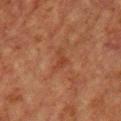Part of a total-body skin-imaging series; this lesion was reviewed on a skin check and was not flagged for biopsy. This is a cross-polarized tile. Longest diameter approximately 3 mm. A male patient, about 60 years old. Located on the chest. A 15 mm close-up tile from a total-body photography series done for melanoma screening. The lesion-visualizer software estimated an area of roughly 4 mm² and a symmetry-axis asymmetry near 0.6. It also reported an average lesion color of about L≈36 a*≈23 b*≈29 (CIELAB) and roughly 5 lightness units darker than nearby skin. The analysis additionally found a border-irregularity index near 6/10, internal color variation of about 1 on a 0–10 scale, and radial color variation of about 0.5. And it measured a nevus-likeness score of about 0/100 and a detector confidence of about 100 out of 100 that the crop contains a lesion.